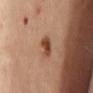Part of a total-body skin-imaging series; this lesion was reviewed on a skin check and was not flagged for biopsy. A 15 mm close-up tile from a total-body photography series done for melanoma screening. Automated image analysis of the tile measured an area of roughly 5 mm², an eccentricity of roughly 0.7, and two-axis asymmetry of about 0.2. About 2.5 mm across. A female patient approximately 60 years of age. Located on the chest.